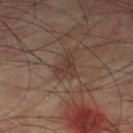illumination = cross-polarized illumination | location = the right thigh | patient = male, in their mid- to late 70s | automated metrics = border irregularity of about 5.5 on a 0–10 scale, internal color variation of about 1.5 on a 0–10 scale, and peripheral color asymmetry of about 0.5; an automated nevus-likeness rating near 10 out of 100 and a detector confidence of about 100 out of 100 that the crop contains a lesion | size = ≈3.5 mm | imaging modality = total-body-photography crop, ~15 mm field of view.